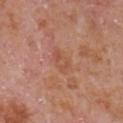Findings:
• notes: catalogued during a skin exam; not biopsied
• lighting: white-light
• body site: the chest
• image source: ~15 mm tile from a whole-body skin photo
• patient: male, aged around 65
• lesion size: ~3 mm (longest diameter)